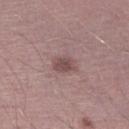Case summary:
- follow-up · no biopsy performed (imaged during a skin exam)
- imaging modality · ~15 mm tile from a whole-body skin photo
- patient · male, aged around 30
- illumination · white-light